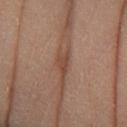Assessment:
Recorded during total-body skin imaging; not selected for excision or biopsy.
Acquisition and patient details:
The lesion-visualizer software estimated a border-irregularity index near 5/10, internal color variation of about 0.5 on a 0–10 scale, and radial color variation of about 0. The lesion is located on the left lower leg. The recorded lesion diameter is about 2.5 mm. Captured under cross-polarized illumination. A female subject, in their 70s. This image is a 15 mm lesion crop taken from a total-body photograph.